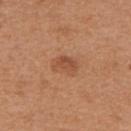The lesion was photographed on a routine skin check and not biopsied; there is no pathology result. The tile uses white-light illumination. A female subject aged around 40. The lesion is on the upper back. Longest diameter approximately 3 mm. A 15 mm close-up tile from a total-body photography series done for melanoma screening.